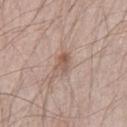– biopsy status · imaged on a skin check; not biopsied
– automated metrics · an area of roughly 4.5 mm², an eccentricity of roughly 0.8, and a shape-asymmetry score of about 0.25 (0 = symmetric); a border-irregularity rating of about 3/10, a color-variation rating of about 4/10, and peripheral color asymmetry of about 1; an automated nevus-likeness rating near 20 out of 100
– body site · the left thigh
– lesion size · ≈3 mm
– subject · male, approximately 40 years of age
– image · ~15 mm tile from a whole-body skin photo
– illumination · white-light